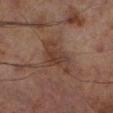Assessment: Part of a total-body skin-imaging series; this lesion was reviewed on a skin check and was not flagged for biopsy. Background: Automated image analysis of the tile measured an average lesion color of about L≈30 a*≈15 b*≈21 (CIELAB), roughly 6 lightness units darker than nearby skin, and a normalized border contrast of about 6.5. A male patient about 70 years old. A region of skin cropped from a whole-body photographic capture, roughly 15 mm wide. Imaged with cross-polarized lighting. From the left lower leg.